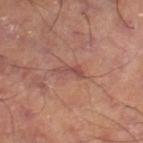Imaged during a routine full-body skin examination; the lesion was not biopsied and no histopathology is available. Measured at roughly 3 mm in maximum diameter. A region of skin cropped from a whole-body photographic capture, roughly 15 mm wide. The total-body-photography lesion software estimated an area of roughly 2.5 mm². The analysis additionally found a mean CIELAB color near L≈49 a*≈25 b*≈25, roughly 8 lightness units darker than nearby skin, and a lesion-to-skin contrast of about 6 (normalized; higher = more distinct). The software also gave border irregularity of about 5.5 on a 0–10 scale and peripheral color asymmetry of about 0. The analysis additionally found a classifier nevus-likeness of about 0/100 and a lesion-detection confidence of about 95/100. The tile uses cross-polarized illumination. From the leg.